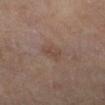biopsy status: catalogued during a skin exam; not biopsied | lighting: cross-polarized illumination | patient: male, in their mid-60s | body site: the left lower leg | image source: ~15 mm tile from a whole-body skin photo.